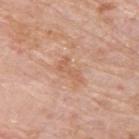Q: Was this lesion biopsied?
A: no biopsy performed (imaged during a skin exam)
Q: Where on the body is the lesion?
A: the upper back
Q: What is the imaging modality?
A: ~15 mm crop, total-body skin-cancer survey
Q: What are the patient's age and sex?
A: male, about 75 years old